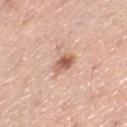Case summary:
• follow-up — imaged on a skin check; not biopsied
• acquisition — ~15 mm tile from a whole-body skin photo
• subject — male, in their 50s
• body site — the left thigh
• lighting — white-light illumination
• lesion diameter — about 2.5 mm